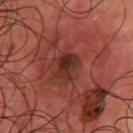– patient: male, approximately 65 years of age
– diameter: ~3.5 mm (longest diameter)
– body site: the chest
– lighting: cross-polarized illumination
– TBP lesion metrics: a lesion area of about 6.5 mm² and an eccentricity of roughly 0.7; an automated nevus-likeness rating near 0 out of 100 and lesion-presence confidence of about 100/100
– image: total-body-photography crop, ~15 mm field of view